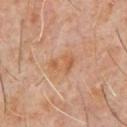The lesion was photographed on a routine skin check and not biopsied; there is no pathology result. Located on the chest. Cropped from a whole-body photographic skin survey; the tile spans about 15 mm. Approximately 3.5 mm at its widest. The tile uses cross-polarized illumination. A male patient, approximately 60 years of age.